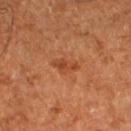workup: imaged on a skin check; not biopsied
tile lighting: cross-polarized illumination
subject: approximately 65 years of age
image-analysis metrics: an average lesion color of about L≈46 a*≈28 b*≈38 (CIELAB), about 8 CIELAB-L* units darker than the surrounding skin, and a normalized border contrast of about 6.5; border irregularity of about 3 on a 0–10 scale, internal color variation of about 3.5 on a 0–10 scale, and a peripheral color-asymmetry measure near 1; a nevus-likeness score of about 10/100 and lesion-presence confidence of about 100/100
image source: 15 mm crop, total-body photography
lesion size: ≈3.5 mm
site: the left lower leg